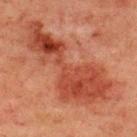Notes:
* follow-up — imaged on a skin check; not biopsied
* subject — male, about 60 years old
* lesion size — ≈12.5 mm
* illumination — cross-polarized illumination
* imaging modality — 15 mm crop, total-body photography
* location — the upper back